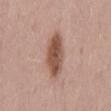Impression: The lesion was photographed on a routine skin check and not biopsied; there is no pathology result.